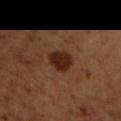This lesion was catalogued during total-body skin photography and was not selected for biopsy.
A 15 mm crop from a total-body photograph taken for skin-cancer surveillance.
This is a cross-polarized tile.
The lesion is on the chest.
About 3.5 mm across.
A male patient aged 48 to 52.